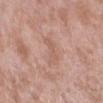follow-up = no biopsy performed (imaged during a skin exam) | location = the right upper arm | tile lighting = white-light illumination | acquisition = ~15 mm crop, total-body skin-cancer survey | lesion diameter = ~3.5 mm (longest diameter) | subject = male, aged around 55.